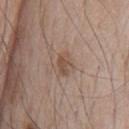workup = imaged on a skin check; not biopsied
lesion size = about 2.5 mm
patient = male, aged 63–67
acquisition = 15 mm crop, total-body photography
anatomic site = the chest
automated lesion analysis = an area of roughly 4 mm² and a shape eccentricity near 0.7; border irregularity of about 3 on a 0–10 scale, a within-lesion color-variation index near 2/10, and radial color variation of about 0.5